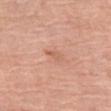| feature | finding |
|---|---|
| notes | total-body-photography surveillance lesion; no biopsy |
| image | 15 mm crop, total-body photography |
| diameter | about 2.5 mm |
| illumination | white-light |
| patient | female, aged approximately 65 |
| anatomic site | the left thigh |